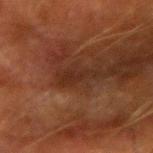{
  "biopsy_status": "not biopsied; imaged during a skin examination",
  "site": "left forearm",
  "patient": {
    "sex": "male",
    "age_approx": 65
  },
  "image": {
    "source": "total-body photography crop",
    "field_of_view_mm": 15
  },
  "lesion_size": {
    "long_diameter_mm_approx": 4.0
  },
  "automated_metrics": {
    "area_mm2_approx": 4.0,
    "shape_asymmetry": 0.45,
    "border_irregularity_0_10": 6.0,
    "color_variation_0_10": 1.0,
    "peripheral_color_asymmetry": 0.5,
    "lesion_detection_confidence_0_100": 70
  },
  "lighting": "cross-polarized"
}